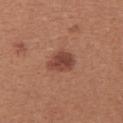{"biopsy_status": "not biopsied; imaged during a skin examination", "image": {"source": "total-body photography crop", "field_of_view_mm": 15}, "site": "back", "automated_metrics": {"eccentricity": 0.65, "shape_asymmetry": 0.2, "vs_skin_darker_L": 11.0, "border_irregularity_0_10": 2.5, "color_variation_0_10": 3.5, "peripheral_color_asymmetry": 1.0}, "patient": {"sex": "female", "age_approx": 40}, "lesion_size": {"long_diameter_mm_approx": 3.5}}